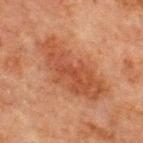Image and clinical context: The lesion is located on the chest. A male subject, aged around 65. Automated image analysis of the tile measured an area of roughly 26 mm², an outline eccentricity of about 0.9 (0 = round, 1 = elongated), and a shape-asymmetry score of about 0.3 (0 = symmetric). The analysis additionally found an average lesion color of about L≈41 a*≈23 b*≈30 (CIELAB) and a normalized lesion–skin contrast near 7. It also reported a border-irregularity rating of about 5/10 and radial color variation of about 1. The software also gave a detector confidence of about 100 out of 100 that the crop contains a lesion. Cropped from a whole-body photographic skin survey; the tile spans about 15 mm.